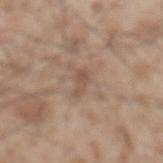Impression:
Captured during whole-body skin photography for melanoma surveillance; the lesion was not biopsied.
Acquisition and patient details:
The total-body-photography lesion software estimated an area of roughly 3 mm² and an eccentricity of roughly 0.9. The analysis additionally found a lesion color around L≈52 a*≈16 b*≈27 in CIELAB, roughly 8 lightness units darker than nearby skin, and a normalized border contrast of about 5.5. Captured under white-light illumination. Longest diameter approximately 3 mm. A male subject, roughly 55 years of age. A lesion tile, about 15 mm wide, cut from a 3D total-body photograph. Located on the abdomen.